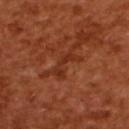The lesion was photographed on a routine skin check and not biopsied; there is no pathology result. A female patient, in their mid-50s. A 15 mm close-up extracted from a 3D total-body photography capture. The lesion is on the upper back.